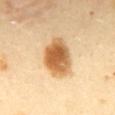{"biopsy_status": "not biopsied; imaged during a skin examination"}